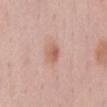Assessment: Part of a total-body skin-imaging series; this lesion was reviewed on a skin check and was not flagged for biopsy. Background: An algorithmic analysis of the crop reported a lesion area of about 4 mm², an outline eccentricity of about 0.75 (0 = round, 1 = elongated), and a symmetry-axis asymmetry near 0.25. And it measured an average lesion color of about L≈60 a*≈23 b*≈29 (CIELAB) and a normalized border contrast of about 6.5. The software also gave a border-irregularity rating of about 2.5/10, a within-lesion color-variation index near 3/10, and radial color variation of about 1. The analysis additionally found a nevus-likeness score of about 75/100. A region of skin cropped from a whole-body photographic capture, roughly 15 mm wide. A male patient, about 60 years old. Captured under white-light illumination. The lesion is on the chest. The recorded lesion diameter is about 2.5 mm.